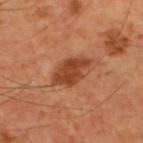notes — total-body-photography surveillance lesion; no biopsy
patient — male, aged 58 to 62
tile lighting — cross-polarized
imaging modality — ~15 mm crop, total-body skin-cancer survey
lesion diameter — ~5 mm (longest diameter)
location — the upper back